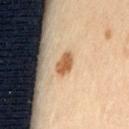follow-up: imaged on a skin check; not biopsied
tile lighting: cross-polarized
image source: 15 mm crop, total-body photography
lesion size: about 3 mm
subject: aged approximately 55
anatomic site: the left thigh
automated lesion analysis: a shape eccentricity near 0.8 and a shape-asymmetry score of about 0.2 (0 = symmetric); border irregularity of about 1.5 on a 0–10 scale, a within-lesion color-variation index near 2.5/10, and a peripheral color-asymmetry measure near 1